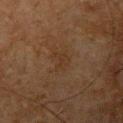Captured during whole-body skin photography for melanoma surveillance; the lesion was not biopsied. Located on the left upper arm. A 15 mm close-up extracted from a 3D total-body photography capture. A male patient, roughly 65 years of age.